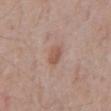The lesion was photographed on a routine skin check and not biopsied; there is no pathology result. From the abdomen. A male subject about 70 years old. The lesion-visualizer software estimated two-axis asymmetry of about 0.25. The recorded lesion diameter is about 2.5 mm. A 15 mm close-up tile from a total-body photography series done for melanoma screening.